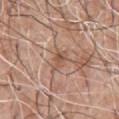Captured during whole-body skin photography for melanoma surveillance; the lesion was not biopsied. The lesion-visualizer software estimated a peripheral color-asymmetry measure near 1. The software also gave an automated nevus-likeness rating near 0 out of 100. A 15 mm crop from a total-body photograph taken for skin-cancer surveillance. The lesion is on the chest. A male subject, aged 58–62. The lesion's longest dimension is about 2.5 mm.